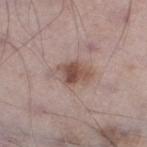Imaged during a routine full-body skin examination; the lesion was not biopsied and no histopathology is available. The lesion's longest dimension is about 5 mm. The lesion is located on the left lower leg. A 15 mm close-up tile from a total-body photography series done for melanoma screening. The subject is a male aged around 70.